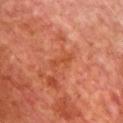follow-up: catalogued during a skin exam; not biopsied | lesion diameter: ~3 mm (longest diameter) | TBP lesion metrics: a lesion area of about 3 mm², an outline eccentricity of about 0.95 (0 = round, 1 = elongated), and two-axis asymmetry of about 0.3; a lesion color around L≈50 a*≈32 b*≈39 in CIELAB, about 6 CIELAB-L* units darker than the surrounding skin, and a lesion-to-skin contrast of about 5.5 (normalized; higher = more distinct) | site: the chest | image: ~15 mm tile from a whole-body skin photo | patient: male, roughly 70 years of age | tile lighting: cross-polarized illumination.